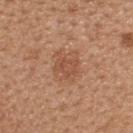Part of a total-body skin-imaging series; this lesion was reviewed on a skin check and was not flagged for biopsy. A 15 mm close-up extracted from a 3D total-body photography capture. A female patient approximately 45 years of age. The total-body-photography lesion software estimated a mean CIELAB color near L≈51 a*≈23 b*≈33, about 7 CIELAB-L* units darker than the surrounding skin, and a normalized lesion–skin contrast near 5.5. Longest diameter approximately 2.5 mm. Located on the upper back. Captured under white-light illumination.